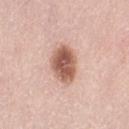Impression:
Part of a total-body skin-imaging series; this lesion was reviewed on a skin check and was not flagged for biopsy.
Acquisition and patient details:
The subject is a female approximately 65 years of age. The recorded lesion diameter is about 4.5 mm. Captured under white-light illumination. This image is a 15 mm lesion crop taken from a total-body photograph. The lesion-visualizer software estimated a classifier nevus-likeness of about 95/100 and a detector confidence of about 100 out of 100 that the crop contains a lesion. From the left thigh.